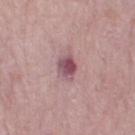- workup — catalogued during a skin exam; not biopsied
- acquisition — ~15 mm tile from a whole-body skin photo
- patient — female, in their mid- to late 60s
- tile lighting — white-light
- anatomic site — the left thigh